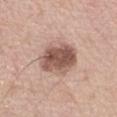Approximately 5 mm at its widest.
A 15 mm crop from a total-body photograph taken for skin-cancer surveillance.
This is a white-light tile.
On the left upper arm.
The patient is a female roughly 50 years of age.